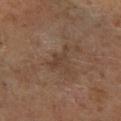follow-up: no biopsy performed (imaged during a skin exam); lighting: cross-polarized; lesion size: about 3.5 mm; image source: total-body-photography crop, ~15 mm field of view; subject: male, approximately 60 years of age; body site: the right leg.